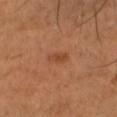Captured during whole-body skin photography for melanoma surveillance; the lesion was not biopsied. A male patient, aged 48 to 52. Automated image analysis of the tile measured an area of roughly 3.5 mm², an outline eccentricity of about 0.85 (0 = round, 1 = elongated), and a shape-asymmetry score of about 0.2 (0 = symmetric). And it measured a border-irregularity rating of about 2/10, a color-variation rating of about 2.5/10, and peripheral color asymmetry of about 1. It also reported a classifier nevus-likeness of about 45/100 and a detector confidence of about 100 out of 100 that the crop contains a lesion. Captured under cross-polarized illumination. About 3 mm across. A 15 mm close-up tile from a total-body photography series done for melanoma screening. On the head or neck.